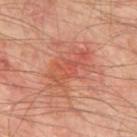Q: How was this image acquired?
A: total-body-photography crop, ~15 mm field of view
Q: Automated lesion metrics?
A: a lesion area of about 9.5 mm² and an eccentricity of roughly 0.9; a lesion color around L≈53 a*≈30 b*≈33 in CIELAB, about 7 CIELAB-L* units darker than the surrounding skin, and a lesion-to-skin contrast of about 5 (normalized; higher = more distinct); a border-irregularity index near 6/10, a color-variation rating of about 3/10, and a peripheral color-asymmetry measure near 0.5
Q: Lesion size?
A: ≈5.5 mm
Q: Illumination type?
A: cross-polarized illumination
Q: What are the patient's age and sex?
A: male, aged 63–67
Q: Lesion location?
A: the mid back